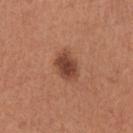follow-up: total-body-photography surveillance lesion; no biopsy | image: ~15 mm tile from a whole-body skin photo | patient: female, roughly 55 years of age | size: about 3.5 mm | illumination: white-light | anatomic site: the left upper arm.